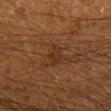| key | value |
|---|---|
| follow-up | no biopsy performed (imaged during a skin exam) |
| illumination | cross-polarized |
| patient | male, aged 58 to 62 |
| anatomic site | the right lower leg |
| acquisition | ~15 mm crop, total-body skin-cancer survey |